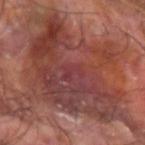Impression:
Part of a total-body skin-imaging series; this lesion was reviewed on a skin check and was not flagged for biopsy.
Background:
Automated tile analysis of the lesion measured a lesion area of about 80 mm², a shape eccentricity near 0.75, and a symmetry-axis asymmetry near 0.35. The software also gave a mean CIELAB color near L≈39 a*≈25 b*≈23 and a normalized lesion–skin contrast near 8. It also reported a border-irregularity rating of about 7.5/10, internal color variation of about 8.5 on a 0–10 scale, and peripheral color asymmetry of about 3. Longest diameter approximately 14 mm. A male patient aged around 60. On the left forearm. A 15 mm close-up extracted from a 3D total-body photography capture. This is a cross-polarized tile.